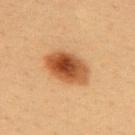Captured during whole-body skin photography for melanoma surveillance; the lesion was not biopsied. The lesion is located on the upper back. This is a cross-polarized tile. A female patient aged around 40. The total-body-photography lesion software estimated an average lesion color of about L≈45 a*≈23 b*≈36 (CIELAB) and a lesion–skin lightness drop of about 15. And it measured a border-irregularity index near 1.5/10, a color-variation rating of about 7.5/10, and radial color variation of about 2. It also reported a classifier nevus-likeness of about 100/100. A close-up tile cropped from a whole-body skin photograph, about 15 mm across.